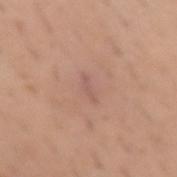A close-up tile cropped from a whole-body skin photograph, about 15 mm across.
The lesion is located on the left forearm.
A male patient about 40 years old.
The recorded lesion diameter is about 2.5 mm.